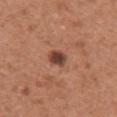follow-up: total-body-photography surveillance lesion; no biopsy
patient: female, aged around 55
acquisition: 15 mm crop, total-body photography
diameter: about 3.5 mm
image-analysis metrics: an area of roughly 7 mm², an eccentricity of roughly 0.55, and two-axis asymmetry of about 0.2; a mean CIELAB color near L≈47 a*≈23 b*≈29, roughly 10 lightness units darker than nearby skin, and a lesion-to-skin contrast of about 7.5 (normalized; higher = more distinct); a border-irregularity index near 2/10 and a peripheral color-asymmetry measure near 1.5; a classifier nevus-likeness of about 65/100 and a lesion-detection confidence of about 100/100
anatomic site: the right upper arm